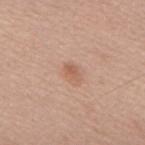| field | value |
|---|---|
| site | the mid back |
| patient | male, aged 68–72 |
| tile lighting | white-light illumination |
| automated lesion analysis | a footprint of about 3 mm², a shape eccentricity near 0.8, and a shape-asymmetry score of about 0.2 (0 = symmetric); a lesion color around L≈59 a*≈21 b*≈31 in CIELAB, roughly 8 lightness units darker than nearby skin, and a normalized border contrast of about 6; a border-irregularity rating of about 1.5/10, internal color variation of about 1.5 on a 0–10 scale, and peripheral color asymmetry of about 0.5 |
| size | ≈2.5 mm |
| imaging modality | 15 mm crop, total-body photography |